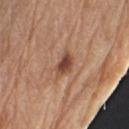Clinical summary:
A male patient, aged 68–72. An algorithmic analysis of the crop reported border irregularity of about 2.5 on a 0–10 scale, a color-variation rating of about 4/10, and peripheral color asymmetry of about 1.5. Longest diameter approximately 3 mm. A 15 mm close-up tile from a total-body photography series done for melanoma screening. On the arm.